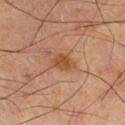Impression:
Imaged during a routine full-body skin examination; the lesion was not biopsied and no histopathology is available.
Acquisition and patient details:
Imaged with cross-polarized lighting. Located on the left lower leg. A 15 mm close-up extracted from a 3D total-body photography capture. A male subject roughly 60 years of age. Automated image analysis of the tile measured a border-irregularity index near 2/10, a color-variation rating of about 2/10, and peripheral color asymmetry of about 0.5.